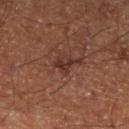biopsy status=imaged on a skin check; not biopsied | body site=the left lower leg | illumination=cross-polarized illumination | image=~15 mm crop, total-body skin-cancer survey | lesion size=~2.5 mm (longest diameter) | subject=male, aged approximately 60.